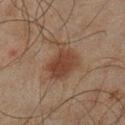| field | value |
|---|---|
| biopsy status | total-body-photography surveillance lesion; no biopsy |
| image source | ~15 mm crop, total-body skin-cancer survey |
| subject | male, about 45 years old |
| body site | the left lower leg |